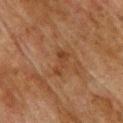Clinical summary: The recorded lesion diameter is about 3 mm. The lesion is located on the upper back. Imaged with cross-polarized lighting. A 15 mm crop from a total-body photograph taken for skin-cancer surveillance. The subject is a male aged around 75.